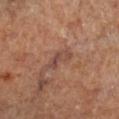Q: Is there a histopathology result?
A: catalogued during a skin exam; not biopsied
Q: What is the anatomic site?
A: the right lower leg
Q: What lighting was used for the tile?
A: cross-polarized illumination
Q: What is the imaging modality?
A: ~15 mm tile from a whole-body skin photo
Q: Who is the patient?
A: male, in their 60s
Q: Lesion size?
A: ≈4 mm